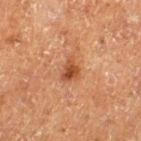The lesion was tiled from a total-body skin photograph and was not biopsied. Cropped from a whole-body photographic skin survey; the tile spans about 15 mm. A male subject aged 73–77. About 3 mm across. Captured under cross-polarized illumination. On the left thigh.